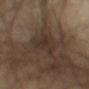Imaged with cross-polarized lighting.
The recorded lesion diameter is about 3.5 mm.
Cropped from a whole-body photographic skin survey; the tile spans about 15 mm.
A male patient aged approximately 60.
On the chest.